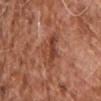The subject is a male about 75 years old.
A 15 mm close-up tile from a total-body photography series done for melanoma screening.
Captured under white-light illumination.
Longest diameter approximately 4 mm.
Located on the chest.